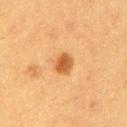About 2.5 mm across.
The subject is a male roughly 40 years of age.
This image is a 15 mm lesion crop taken from a total-body photograph.
This is a cross-polarized tile.
From the arm.